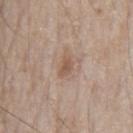| feature | finding |
|---|---|
| follow-up | no biopsy performed (imaged during a skin exam) |
| patient | male, aged 78–82 |
| anatomic site | the chest |
| lighting | white-light |
| image | ~15 mm tile from a whole-body skin photo |